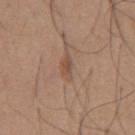| key | value |
|---|---|
| TBP lesion metrics | a lesion color around L≈50 a*≈19 b*≈29 in CIELAB and a normalized lesion–skin contrast near 6 |
| lesion diameter | ≈3 mm |
| patient | male, about 65 years old |
| imaging modality | ~15 mm tile from a whole-body skin photo |
| tile lighting | white-light |
| body site | the abdomen |